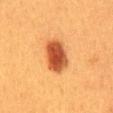The lesion was photographed on a routine skin check and not biopsied; there is no pathology result. The lesion-visualizer software estimated a mean CIELAB color near L≈44 a*≈27 b*≈37, roughly 16 lightness units darker than nearby skin, and a normalized lesion–skin contrast near 11.5. And it measured border irregularity of about 2 on a 0–10 scale and internal color variation of about 4.5 on a 0–10 scale. It also reported an automated nevus-likeness rating near 100 out of 100 and a lesion-detection confidence of about 100/100. A 15 mm close-up tile from a total-body photography series done for melanoma screening. A female subject aged approximately 40. Longest diameter approximately 5 mm. The tile uses cross-polarized illumination. The lesion is located on the mid back.